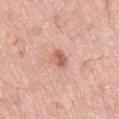workup: no biopsy performed (imaged during a skin exam)
image source: ~15 mm tile from a whole-body skin photo
body site: the right thigh
tile lighting: white-light
subject: male, about 80 years old